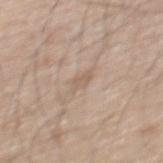  image:
    source: total-body photography crop
    field_of_view_mm: 15
  lesion_size:
    long_diameter_mm_approx: 3.0
  lighting: white-light
  patient:
    sex: male
    age_approx: 65
  site: mid back